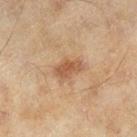| key | value |
|---|---|
| follow-up | imaged on a skin check; not biopsied |
| imaging modality | total-body-photography crop, ~15 mm field of view |
| diameter | ~3.5 mm (longest diameter) |
| patient | female, in their 60s |
| body site | the right lower leg |
| automated metrics | a mean CIELAB color near L≈50 a*≈19 b*≈32, a lesion–skin lightness drop of about 10, and a normalized lesion–skin contrast near 7; a border-irregularity rating of about 3/10 and peripheral color asymmetry of about 1 |
| tile lighting | cross-polarized |